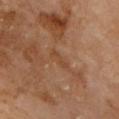notes: imaged on a skin check; not biopsied
imaging modality: ~15 mm tile from a whole-body skin photo
patient: male, about 70 years old
site: the front of the torso